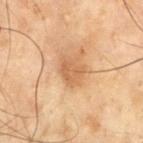biopsy status: no biopsy performed (imaged during a skin exam); lesion size: ~3 mm (longest diameter); acquisition: ~15 mm tile from a whole-body skin photo; patient: male, aged 68–72; body site: the chest.